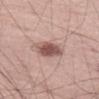{"biopsy_status": "not biopsied; imaged during a skin examination", "patient": {"sex": "male", "age_approx": 55}, "lesion_size": {"long_diameter_mm_approx": 3.5}, "image": {"source": "total-body photography crop", "field_of_view_mm": 15}, "lighting": "white-light", "site": "leg"}